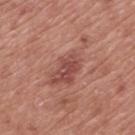The subject is a male aged approximately 75. From the mid back. This image is a 15 mm lesion crop taken from a total-body photograph. Longest diameter approximately 7.5 mm.